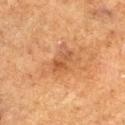Clinical impression: Imaged during a routine full-body skin examination; the lesion was not biopsied and no histopathology is available. Context: The lesion is on the right thigh. A 15 mm crop from a total-body photograph taken for skin-cancer surveillance. Imaged with cross-polarized lighting. Approximately 3 mm at its widest. A male subject, in their mid-70s.